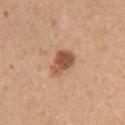Captured during whole-body skin photography for melanoma surveillance; the lesion was not biopsied. Located on the arm. The subject is a female in their 30s. Captured under white-light illumination. The lesion-visualizer software estimated a lesion area of about 7.5 mm², an outline eccentricity of about 0.75 (0 = round, 1 = elongated), and two-axis asymmetry of about 0.3. The analysis additionally found border irregularity of about 2.5 on a 0–10 scale, a color-variation rating of about 5/10, and a peripheral color-asymmetry measure near 1.5. The software also gave a classifier nevus-likeness of about 80/100 and lesion-presence confidence of about 100/100. Approximately 3.5 mm at its widest. A 15 mm close-up extracted from a 3D total-body photography capture.